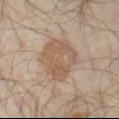This lesion was catalogued during total-body skin photography and was not selected for biopsy. A male patient, in their mid- to late 60s. On the right thigh. A 15 mm crop from a total-body photograph taken for skin-cancer surveillance.